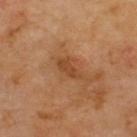{"biopsy_status": "not biopsied; imaged during a skin examination", "lesion_size": {"long_diameter_mm_approx": 3.0}, "automated_metrics": {"area_mm2_approx": 4.0, "eccentricity": 0.9, "shape_asymmetry": 0.35, "cielab_L": 44, "cielab_a": 23, "cielab_b": 36, "vs_skin_contrast_norm": 6.5, "nevus_likeness_0_100": 10, "lesion_detection_confidence_0_100": 100}, "image": {"source": "total-body photography crop", "field_of_view_mm": 15}, "site": "upper back", "patient": {"sex": "male", "age_approx": 70}, "lighting": "cross-polarized"}